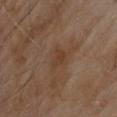follow-up=no biopsy performed (imaged during a skin exam) | subject=male, aged approximately 65 | site=the upper back | TBP lesion metrics=a lesion color around L≈38 a*≈18 b*≈29 in CIELAB, roughly 6 lightness units darker than nearby skin, and a normalized border contrast of about 6; a border-irregularity rating of about 3.5/10, a within-lesion color-variation index near 1.5/10, and radial color variation of about 0.5; a nevus-likeness score of about 0/100 and a detector confidence of about 100 out of 100 that the crop contains a lesion | imaging modality=total-body-photography crop, ~15 mm field of view | tile lighting=cross-polarized | size=~2.5 mm (longest diameter).